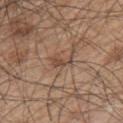Part of a total-body skin-imaging series; this lesion was reviewed on a skin check and was not flagged for biopsy.
A male subject, aged approximately 50.
The lesion is on the back.
A 15 mm crop from a total-body photograph taken for skin-cancer surveillance.
The recorded lesion diameter is about 3 mm.